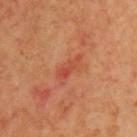{
  "biopsy_status": "not biopsied; imaged during a skin examination",
  "patient": {
    "sex": "male",
    "age_approx": 70
  },
  "lesion_size": {
    "long_diameter_mm_approx": 4.0
  },
  "image": {
    "source": "total-body photography crop",
    "field_of_view_mm": 15
  },
  "site": "chest",
  "lighting": "cross-polarized"
}